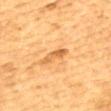biopsy status = imaged on a skin check; not biopsied
site = the upper back
image = 15 mm crop, total-body photography
tile lighting = cross-polarized illumination
subject = male, about 85 years old or older
lesion diameter = about 3.5 mm